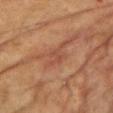Part of a total-body skin-imaging series; this lesion was reviewed on a skin check and was not flagged for biopsy.
The tile uses cross-polarized illumination.
This image is a 15 mm lesion crop taken from a total-body photograph.
The subject is a female about 60 years old.
On the chest.
Approximately 2.5 mm at its widest.